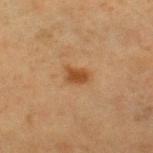Impression: The lesion was photographed on a routine skin check and not biopsied; there is no pathology result. Image and clinical context: This image is a 15 mm lesion crop taken from a total-body photograph. A female subject, about 40 years old. Captured under cross-polarized illumination. Automated image analysis of the tile measured an area of roughly 4.5 mm², an eccentricity of roughly 0.8, and two-axis asymmetry of about 0.25. The analysis additionally found a classifier nevus-likeness of about 95/100 and lesion-presence confidence of about 100/100. The lesion is located on the leg. The lesion's longest dimension is about 3 mm.